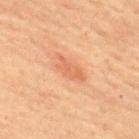Q: Automated lesion metrics?
A: a shape eccentricity near 0.9 and a symmetry-axis asymmetry near 0.35; a mean CIELAB color near L≈53 a*≈23 b*≈33, a lesion–skin lightness drop of about 7, and a normalized border contrast of about 5.5; border irregularity of about 4 on a 0–10 scale and peripheral color asymmetry of about 1; a detector confidence of about 100 out of 100 that the crop contains a lesion
Q: Illumination type?
A: cross-polarized illumination
Q: Patient demographics?
A: male, in their 60s
Q: Lesion location?
A: the upper back
Q: What kind of image is this?
A: ~15 mm crop, total-body skin-cancer survey